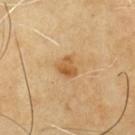The lesion was tiled from a total-body skin photograph and was not biopsied.
The lesion-visualizer software estimated an automated nevus-likeness rating near 65 out of 100.
Captured under cross-polarized illumination.
A 15 mm close-up tile from a total-body photography series done for melanoma screening.
About 2.5 mm across.
On the front of the torso.
The patient is a male aged around 65.